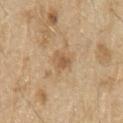{"biopsy_status": "not biopsied; imaged during a skin examination", "patient": {"sex": "male", "age_approx": 70}, "image": {"source": "total-body photography crop", "field_of_view_mm": 15}, "automated_metrics": {"cielab_L": 57, "cielab_a": 17, "cielab_b": 36, "vs_skin_darker_L": 9.0, "vs_skin_contrast_norm": 6.0, "border_irregularity_0_10": 3.0, "color_variation_0_10": 2.5, "peripheral_color_asymmetry": 1.0, "nevus_likeness_0_100": 10}, "lighting": "white-light", "site": "right upper arm"}